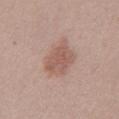Background: The tile uses white-light illumination. The lesion is on the abdomen. A male subject aged 38–42. Cropped from a total-body skin-imaging series; the visible field is about 15 mm. About 5.5 mm across.